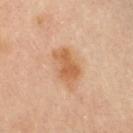Case summary:
* follow-up — total-body-photography surveillance lesion; no biopsy
* patient — female, roughly 50 years of age
* imaging modality — total-body-photography crop, ~15 mm field of view
* size — ~4.5 mm (longest diameter)
* site — the back
* automated lesion analysis — an eccentricity of roughly 0.8; a border-irregularity rating of about 2.5/10 and a color-variation rating of about 3.5/10